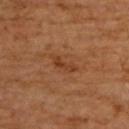notes: no biopsy performed (imaged during a skin exam)
acquisition: total-body-photography crop, ~15 mm field of view
diameter: ~3 mm (longest diameter)
subject: male, aged around 65
automated metrics: a lesion area of about 3.5 mm² and a symmetry-axis asymmetry near 0.45; a mean CIELAB color near L≈38 a*≈23 b*≈34 and a lesion-to-skin contrast of about 6.5 (normalized; higher = more distinct); a color-variation rating of about 2/10 and a peripheral color-asymmetry measure near 0.5
location: the upper back
lighting: cross-polarized